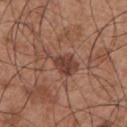The lesion was photographed on a routine skin check and not biopsied; there is no pathology result. Measured at roughly 5 mm in maximum diameter. Located on the chest. A male subject about 55 years old. Cropped from a whole-body photographic skin survey; the tile spans about 15 mm. The tile uses white-light illumination. Automated tile analysis of the lesion measured a footprint of about 7.5 mm², an outline eccentricity of about 0.85 (0 = round, 1 = elongated), and a symmetry-axis asymmetry near 0.5.